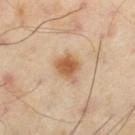Q: Is there a histopathology result?
A: total-body-photography surveillance lesion; no biopsy
Q: What is the imaging modality?
A: total-body-photography crop, ~15 mm field of view
Q: What lighting was used for the tile?
A: cross-polarized
Q: What did automated image analysis measure?
A: a classifier nevus-likeness of about 95/100 and a lesion-detection confidence of about 100/100
Q: How large is the lesion?
A: about 3.5 mm
Q: Patient demographics?
A: male, aged 53–57
Q: What is the anatomic site?
A: the left thigh